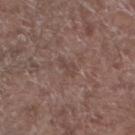biopsy status=no biopsy performed (imaged during a skin exam).